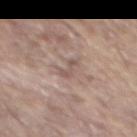Captured during whole-body skin photography for melanoma surveillance; the lesion was not biopsied.
The lesion is on the back.
A 15 mm close-up tile from a total-body photography series done for melanoma screening.
The recorded lesion diameter is about 3 mm.
Captured under white-light illumination.
A male subject aged 63 to 67.
The lesion-visualizer software estimated a lesion color around L≈54 a*≈16 b*≈22 in CIELAB and a lesion-to-skin contrast of about 5.5 (normalized; higher = more distinct). And it measured a classifier nevus-likeness of about 0/100.